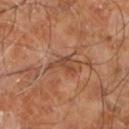Clinical impression:
Captured during whole-body skin photography for melanoma surveillance; the lesion was not biopsied.
Background:
A 15 mm crop from a total-body photograph taken for skin-cancer surveillance. From the right leg. The subject is a male aged 58 to 62. The lesion-visualizer software estimated a footprint of about 5 mm², an eccentricity of roughly 0.85, and two-axis asymmetry of about 0.6. And it measured an average lesion color of about L≈44 a*≈21 b*≈30 (CIELAB) and about 8 CIELAB-L* units darker than the surrounding skin. The analysis additionally found lesion-presence confidence of about 80/100. Captured under cross-polarized illumination.